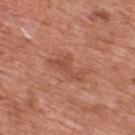Part of a total-body skin-imaging series; this lesion was reviewed on a skin check and was not flagged for biopsy. The lesion is on the upper back. A 15 mm crop from a total-body photograph taken for skin-cancer surveillance. A male patient, roughly 70 years of age.